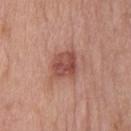Assessment:
This lesion was catalogued during total-body skin photography and was not selected for biopsy.
Acquisition and patient details:
The recorded lesion diameter is about 3.5 mm. Captured under white-light illumination. Cropped from a total-body skin-imaging series; the visible field is about 15 mm. A male subject aged around 55. Automated tile analysis of the lesion measured an average lesion color of about L≈50 a*≈26 b*≈26 (CIELAB), about 11 CIELAB-L* units darker than the surrounding skin, and a normalized lesion–skin contrast near 8. And it measured border irregularity of about 1.5 on a 0–10 scale. And it measured an automated nevus-likeness rating near 85 out of 100 and lesion-presence confidence of about 100/100. From the front of the torso.